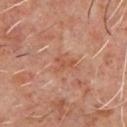• workup · total-body-photography surveillance lesion; no biopsy
• lighting · cross-polarized
• body site · the chest
• imaging modality · ~15 mm tile from a whole-body skin photo
• subject · male, about 60 years old